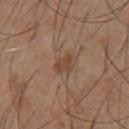follow-up: no biopsy performed (imaged during a skin exam) | image source: ~15 mm tile from a whole-body skin photo | TBP lesion metrics: a mean CIELAB color near L≈45 a*≈19 b*≈29, roughly 8 lightness units darker than nearby skin, and a normalized lesion–skin contrast near 6.5; a nevus-likeness score of about 5/100 and a lesion-detection confidence of about 100/100 | patient: male, aged 53 to 57 | lighting: white-light | location: the chest.